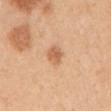Image and clinical context: A female subject aged 43–47. A close-up tile cropped from a whole-body skin photograph, about 15 mm across. On the left upper arm.